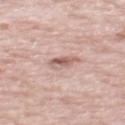{
  "biopsy_status": "not biopsied; imaged during a skin examination",
  "lesion_size": {
    "long_diameter_mm_approx": 3.0
  },
  "automated_metrics": {
    "cielab_L": 60,
    "cielab_a": 20,
    "cielab_b": 24,
    "vs_skin_darker_L": 11.0,
    "vs_skin_contrast_norm": 7.0,
    "nevus_likeness_0_100": 15,
    "lesion_detection_confidence_0_100": 100
  },
  "image": {
    "source": "total-body photography crop",
    "field_of_view_mm": 15
  },
  "site": "back",
  "patient": {
    "sex": "male",
    "age_approx": 70
  }
}